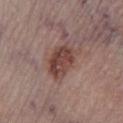Clinical impression: Imaged during a routine full-body skin examination; the lesion was not biopsied and no histopathology is available. Acquisition and patient details: A close-up tile cropped from a whole-body skin photograph, about 15 mm across. The subject is a male aged around 55. The lesion-visualizer software estimated an outline eccentricity of about 0.75 (0 = round, 1 = elongated) and two-axis asymmetry of about 0.15. The analysis additionally found a normalized lesion–skin contrast near 9.5. The analysis additionally found a classifier nevus-likeness of about 70/100. The recorded lesion diameter is about 4.5 mm. The lesion is located on the left lower leg.